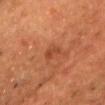Impression: Captured during whole-body skin photography for melanoma surveillance; the lesion was not biopsied. Image and clinical context: The lesion's longest dimension is about 2.5 mm. A lesion tile, about 15 mm wide, cut from a 3D total-body photograph. From the head or neck. This is a cross-polarized tile. A male subject, aged around 50.